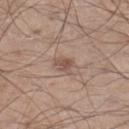follow-up: imaged on a skin check; not biopsied | patient: male, in their mid- to late 50s | size: ≈3 mm | location: the right lower leg | tile lighting: white-light | acquisition: ~15 mm crop, total-body skin-cancer survey.